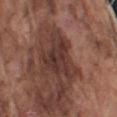Recorded during total-body skin imaging; not selected for excision or biopsy.
A male subject aged 73 to 77.
This image is a 15 mm lesion crop taken from a total-body photograph.
Captured under white-light illumination.
About 6 mm across.
Automated image analysis of the tile measured a lesion area of about 14 mm² and a shape-asymmetry score of about 0.55 (0 = symmetric). The analysis additionally found an average lesion color of about L≈34 a*≈20 b*≈22 (CIELAB), roughly 10 lightness units darker than nearby skin, and a lesion-to-skin contrast of about 9 (normalized; higher = more distinct). And it measured a border-irregularity index near 7/10 and peripheral color asymmetry of about 1.5.
From the left upper arm.